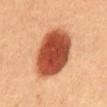This lesion was catalogued during total-body skin photography and was not selected for biopsy. Longest diameter approximately 6.5 mm. A region of skin cropped from a whole-body photographic capture, roughly 15 mm wide. Captured under cross-polarized illumination. From the abdomen. The patient is a female roughly 60 years of age.